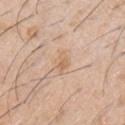| field | value |
|---|---|
| notes | catalogued during a skin exam; not biopsied |
| patient | male, aged around 60 |
| body site | the chest |
| image source | ~15 mm crop, total-body skin-cancer survey |
| image-analysis metrics | about 7 CIELAB-L* units darker than the surrounding skin |